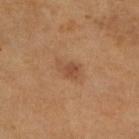Recorded during total-body skin imaging; not selected for excision or biopsy.
From the right lower leg.
Captured under cross-polarized illumination.
This image is a 15 mm lesion crop taken from a total-body photograph.
A female patient approximately 55 years of age.